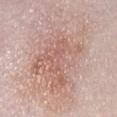Findings:
• notes: catalogued during a skin exam; not biopsied
• TBP lesion metrics: a color-variation rating of about 5.5/10 and peripheral color asymmetry of about 2; an automated nevus-likeness rating near 0 out of 100 and a lesion-detection confidence of about 100/100
• acquisition: ~15 mm crop, total-body skin-cancer survey
• tile lighting: white-light
• anatomic site: the abdomen
• subject: female, roughly 50 years of age
• size: ~11 mm (longest diameter)